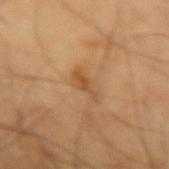This lesion was catalogued during total-body skin photography and was not selected for biopsy.
Imaged with cross-polarized lighting.
The total-body-photography lesion software estimated an area of roughly 3.5 mm², an outline eccentricity of about 0.9 (0 = round, 1 = elongated), and two-axis asymmetry of about 0.4. It also reported a normalized lesion–skin contrast near 6. And it measured a classifier nevus-likeness of about 25/100 and lesion-presence confidence of about 100/100.
From the left forearm.
A lesion tile, about 15 mm wide, cut from a 3D total-body photograph.
A male patient about 60 years old.
About 3 mm across.